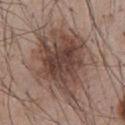Recorded during total-body skin imaging; not selected for excision or biopsy. Automated tile analysis of the lesion measured a lesion area of about 45 mm² and a shape-asymmetry score of about 0.3 (0 = symmetric). The software also gave roughly 11 lightness units darker than nearby skin. The software also gave a border-irregularity index near 5.5/10 and a color-variation rating of about 6/10. It also reported a lesion-detection confidence of about 100/100. On the chest. Imaged with white-light lighting. Approximately 10.5 mm at its widest. A 15 mm close-up extracted from a 3D total-body photography capture. A male subject aged approximately 55.